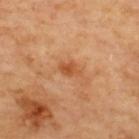Impression:
Part of a total-body skin-imaging series; this lesion was reviewed on a skin check and was not flagged for biopsy.
Image and clinical context:
The lesion-visualizer software estimated a border-irregularity index near 3/10 and peripheral color asymmetry of about 0.5. A 15 mm close-up tile from a total-body photography series done for melanoma screening. Longest diameter approximately 3 mm. On the upper back.